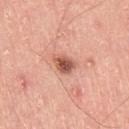The lesion was photographed on a routine skin check and not biopsied; there is no pathology result. On the leg. Captured under white-light illumination. Cropped from a total-body skin-imaging series; the visible field is about 15 mm. Automated image analysis of the tile measured a footprint of about 5.5 mm² and an eccentricity of roughly 0.75. The analysis additionally found about 15 CIELAB-L* units darker than the surrounding skin and a lesion-to-skin contrast of about 9 (normalized; higher = more distinct). And it measured radial color variation of about 1.5. The subject is a male approximately 50 years of age. The lesion's longest dimension is about 3 mm.